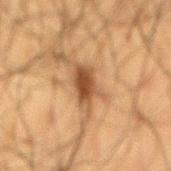workup = total-body-photography surveillance lesion; no biopsy
automated lesion analysis = an average lesion color of about L≈39 a*≈17 b*≈30 (CIELAB) and a normalized lesion–skin contrast near 9.5; border irregularity of about 5.5 on a 0–10 scale, a within-lesion color-variation index near 3/10, and radial color variation of about 1
patient = male, aged approximately 60
image source = total-body-photography crop, ~15 mm field of view
site = the mid back